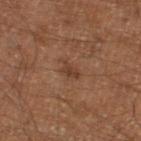follow-up: total-body-photography surveillance lesion; no biopsy
image: ~15 mm tile from a whole-body skin photo
diameter: ≈2.5 mm
illumination: cross-polarized illumination
patient: male, roughly 60 years of age
site: the left lower leg
automated lesion analysis: a shape eccentricity near 0.75